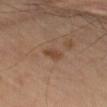The lesion was photographed on a routine skin check and not biopsied; there is no pathology result. About 2.5 mm across. Imaged with cross-polarized lighting. A roughly 15 mm field-of-view crop from a total-body skin photograph. A male patient in their 70s. Located on the left thigh.